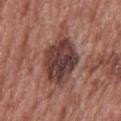follow-up=catalogued during a skin exam; not biopsied
subject=female, roughly 60 years of age
imaging modality=~15 mm crop, total-body skin-cancer survey
diameter=about 6.5 mm
location=the upper back